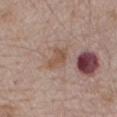Acquisition and patient details: Imaged with white-light lighting. An algorithmic analysis of the crop reported a footprint of about 4.5 mm², an eccentricity of roughly 0.75, and a symmetry-axis asymmetry near 0.4. And it measured a classifier nevus-likeness of about 5/100 and lesion-presence confidence of about 100/100. On the mid back. A 15 mm close-up extracted from a 3D total-body photography capture. The subject is a male aged 63–67.